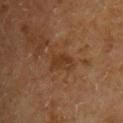<record>
  <patient>
    <sex>male</sex>
    <age_approx>65</age_approx>
  </patient>
  <image>
    <source>total-body photography crop</source>
    <field_of_view_mm>15</field_of_view_mm>
  </image>
  <site>chest</site>
</record>